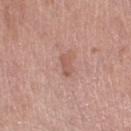Impression: Imaged during a routine full-body skin examination; the lesion was not biopsied and no histopathology is available. Image and clinical context: The total-body-photography lesion software estimated a detector confidence of about 100 out of 100 that the crop contains a lesion. A region of skin cropped from a whole-body photographic capture, roughly 15 mm wide. Captured under white-light illumination. The subject is a female aged 63–67. The lesion is on the right thigh. The recorded lesion diameter is about 2.5 mm.